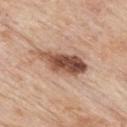biopsy status: total-body-photography surveillance lesion; no biopsy
site: the upper back
image source: total-body-photography crop, ~15 mm field of view
subject: male, approximately 85 years of age or older
image-analysis metrics: a footprint of about 13 mm², a shape eccentricity near 0.9, and two-axis asymmetry of about 0.25; an average lesion color of about L≈51 a*≈21 b*≈29 (CIELAB), about 17 CIELAB-L* units darker than the surrounding skin, and a lesion-to-skin contrast of about 11.5 (normalized; higher = more distinct); border irregularity of about 3.5 on a 0–10 scale, a color-variation rating of about 9/10, and peripheral color asymmetry of about 4; an automated nevus-likeness rating near 95 out of 100 and a detector confidence of about 100 out of 100 that the crop contains a lesion
size: ~6.5 mm (longest diameter)